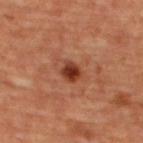Imaged during a routine full-body skin examination; the lesion was not biopsied and no histopathology is available. The lesion is located on the upper back. Imaged with cross-polarized lighting. Automated tile analysis of the lesion measured about 13 CIELAB-L* units darker than the surrounding skin and a lesion-to-skin contrast of about 10 (normalized; higher = more distinct). And it measured border irregularity of about 1.5 on a 0–10 scale, a color-variation rating of about 4/10, and peripheral color asymmetry of about 1. And it measured a classifier nevus-likeness of about 95/100 and a lesion-detection confidence of about 100/100. About 2.5 mm across. A female patient in their mid-40s. Cropped from a total-body skin-imaging series; the visible field is about 15 mm.